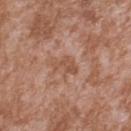Captured during whole-body skin photography for melanoma surveillance; the lesion was not biopsied.
The lesion is located on the upper back.
Longest diameter approximately 3 mm.
A close-up tile cropped from a whole-body skin photograph, about 15 mm across.
A male subject roughly 45 years of age.
The lesion-visualizer software estimated a classifier nevus-likeness of about 0/100 and a detector confidence of about 100 out of 100 that the crop contains a lesion.
This is a white-light tile.